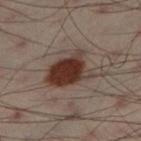Recorded during total-body skin imaging; not selected for excision or biopsy. A roughly 15 mm field-of-view crop from a total-body skin photograph. Located on the left lower leg. A male patient, approximately 50 years of age.